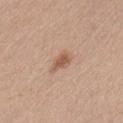Part of a total-body skin-imaging series; this lesion was reviewed on a skin check and was not flagged for biopsy.